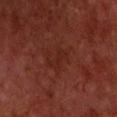{"biopsy_status": "not biopsied; imaged during a skin examination", "site": "chest", "lighting": "cross-polarized", "automated_metrics": {"area_mm2_approx": 4.0, "eccentricity": 0.9, "shape_asymmetry": 0.55, "border_irregularity_0_10": 6.5, "color_variation_0_10": 0.0, "peripheral_color_asymmetry": 0.0}, "lesion_size": {"long_diameter_mm_approx": 3.5}, "patient": {"sex": "male", "age_approx": 60}, "image": {"source": "total-body photography crop", "field_of_view_mm": 15}}